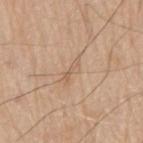No biopsy was performed on this lesion — it was imaged during a full skin examination and was not determined to be concerning.
A male patient, roughly 80 years of age.
On the left arm.
This image is a 15 mm lesion crop taken from a total-body photograph.